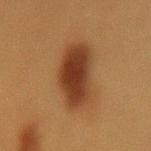Clinical impression: Recorded during total-body skin imaging; not selected for excision or biopsy.